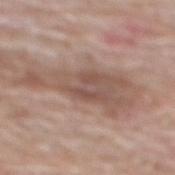* workup: imaged on a skin check; not biopsied
* illumination: white-light
* location: the mid back
* patient: male, in their 80s
* imaging modality: 15 mm crop, total-body photography